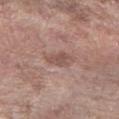No biopsy was performed on this lesion — it was imaged during a full skin examination and was not determined to be concerning. Imaged with white-light lighting. A male patient aged around 70. About 3 mm across. A region of skin cropped from a whole-body photographic capture, roughly 15 mm wide. Located on the left forearm.